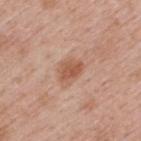{
  "patient": {
    "sex": "male",
    "age_approx": 40
  },
  "site": "upper back",
  "image": {
    "source": "total-body photography crop",
    "field_of_view_mm": 15
  }
}